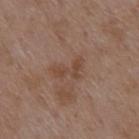The patient is a female aged 68 to 72.
An algorithmic analysis of the crop reported a symmetry-axis asymmetry near 0.5. It also reported a border-irregularity index near 6/10, a color-variation rating of about 1.5/10, and radial color variation of about 0.5. It also reported a classifier nevus-likeness of about 0/100 and lesion-presence confidence of about 100/100.
Imaged with white-light lighting.
A 15 mm close-up tile from a total-body photography series done for melanoma screening.
On the upper back.